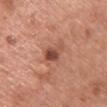| key | value |
|---|---|
| notes | no biopsy performed (imaged during a skin exam) |
| subject | female, roughly 60 years of age |
| lesion size | ~2.5 mm (longest diameter) |
| acquisition | total-body-photography crop, ~15 mm field of view |
| illumination | white-light illumination |
| body site | the chest |